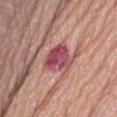Imaged during a routine full-body skin examination; the lesion was not biopsied and no histopathology is available. A female subject, in their mid-70s. Imaged with white-light lighting. A 15 mm close-up tile from a total-body photography series done for melanoma screening. Located on the abdomen.